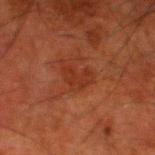Impression: Imaged during a routine full-body skin examination; the lesion was not biopsied and no histopathology is available. Background: The subject is a male aged 78–82. Cropped from a whole-body photographic skin survey; the tile spans about 15 mm. On the left lower leg.